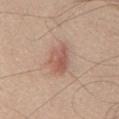Impression: The lesion was tiled from a total-body skin photograph and was not biopsied. Clinical summary: Imaged with white-light lighting. A male subject in their mid-60s. Located on the chest. The recorded lesion diameter is about 4 mm. A 15 mm close-up extracted from a 3D total-body photography capture. The lesion-visualizer software estimated a lesion area of about 7.5 mm², an eccentricity of roughly 0.8, and a symmetry-axis asymmetry near 0.25. The software also gave an average lesion color of about L≈56 a*≈22 b*≈27 (CIELAB), about 10 CIELAB-L* units darker than the surrounding skin, and a normalized border contrast of about 7. The analysis additionally found border irregularity of about 3 on a 0–10 scale, internal color variation of about 4.5 on a 0–10 scale, and peripheral color asymmetry of about 1.5. The software also gave an automated nevus-likeness rating near 50 out of 100 and lesion-presence confidence of about 100/100.